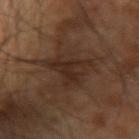Assessment: No biopsy was performed on this lesion — it was imaged during a full skin examination and was not determined to be concerning. Image and clinical context: A 15 mm crop from a total-body photograph taken for skin-cancer surveillance. The patient is a male in their 60s. The lesion is on the right arm. Approximately 4.5 mm at its widest. Captured under cross-polarized illumination. An algorithmic analysis of the crop reported a shape eccentricity near 0.35 and a symmetry-axis asymmetry near 0.65. The analysis additionally found an average lesion color of about L≈25 a*≈15 b*≈22 (CIELAB), about 7 CIELAB-L* units darker than the surrounding skin, and a normalized lesion–skin contrast near 7.5. It also reported a border-irregularity index near 8.5/10, a color-variation rating of about 1.5/10, and radial color variation of about 0.5.